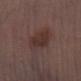Clinical impression:
The lesion was photographed on a routine skin check and not biopsied; there is no pathology result.